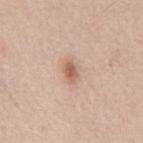Case summary:
- biopsy status: imaged on a skin check; not biopsied
- site: the mid back
- lesion size: ≈2.5 mm
- illumination: white-light
- patient: male, aged 43 to 47
- image source: ~15 mm tile from a whole-body skin photo